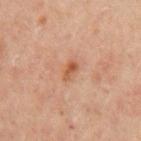Notes:
– notes: total-body-photography surveillance lesion; no biopsy
– acquisition: ~15 mm tile from a whole-body skin photo
– diameter: about 2.5 mm
– location: the arm
– tile lighting: cross-polarized
– patient: male, roughly 45 years of age